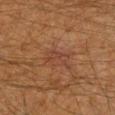{
  "biopsy_status": "not biopsied; imaged during a skin examination",
  "image": {
    "source": "total-body photography crop",
    "field_of_view_mm": 15
  },
  "site": "right forearm",
  "patient": {
    "sex": "male",
    "age_approx": 50
  },
  "lighting": "cross-polarized"
}